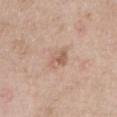The lesion was photographed on a routine skin check and not biopsied; there is no pathology result. On the left upper arm. An algorithmic analysis of the crop reported a footprint of about 5.5 mm² and two-axis asymmetry of about 0.25. The software also gave a border-irregularity rating of about 2.5/10 and radial color variation of about 2. The subject is a male in their 50s. Captured under white-light illumination. A 15 mm close-up extracted from a 3D total-body photography capture.